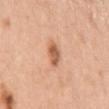| key | value |
|---|---|
| biopsy status | catalogued during a skin exam; not biopsied |
| location | the left upper arm |
| tile lighting | white-light |
| image | ~15 mm crop, total-body skin-cancer survey |
| diameter | ≈3 mm |
| subject | female, approximately 45 years of age |